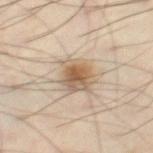The lesion was tiled from a total-body skin photograph and was not biopsied.
Longest diameter approximately 4 mm.
The subject is a male aged 53 to 57.
Automated image analysis of the tile measured a footprint of about 10 mm², an outline eccentricity of about 0.5 (0 = round, 1 = elongated), and a shape-asymmetry score of about 0.25 (0 = symmetric).
The lesion is on the right thigh.
A lesion tile, about 15 mm wide, cut from a 3D total-body photograph.